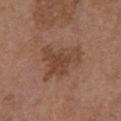{"biopsy_status": "not biopsied; imaged during a skin examination", "image": {"source": "total-body photography crop", "field_of_view_mm": 15}, "automated_metrics": {"eccentricity": 0.65, "shape_asymmetry": 0.6, "color_variation_0_10": 3.5, "peripheral_color_asymmetry": 1.0}, "patient": {"sex": "female", "age_approx": 65}, "lesion_size": {"long_diameter_mm_approx": 5.5}, "site": "front of the torso", "lighting": "white-light"}